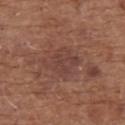Captured during whole-body skin photography for melanoma surveillance; the lesion was not biopsied. Imaged with white-light lighting. The subject is a female roughly 75 years of age. The recorded lesion diameter is about 4 mm. From the upper back. A region of skin cropped from a whole-body photographic capture, roughly 15 mm wide.